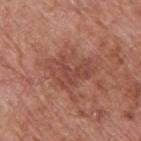{"biopsy_status": "not biopsied; imaged during a skin examination", "lesion_size": {"long_diameter_mm_approx": 5.0}, "automated_metrics": {"eccentricity": 0.7, "shape_asymmetry": 0.35, "border_irregularity_0_10": 5.0, "peripheral_color_asymmetry": 1.0}, "image": {"source": "total-body photography crop", "field_of_view_mm": 15}, "site": "back", "patient": {"sex": "male", "age_approx": 65}, "lighting": "white-light"}